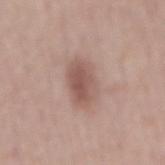workup: catalogued during a skin exam; not biopsied
image: ~15 mm crop, total-body skin-cancer survey
anatomic site: the mid back
subject: male, aged 58–62